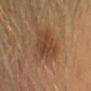Captured during whole-body skin photography for melanoma surveillance; the lesion was not biopsied. This is a cross-polarized tile. A region of skin cropped from a whole-body photographic capture, roughly 15 mm wide. A female subject, aged around 50. The recorded lesion diameter is about 6 mm. Automated tile analysis of the lesion measured a normalized lesion–skin contrast near 7. The analysis additionally found a within-lesion color-variation index near 3.5/10. And it measured a classifier nevus-likeness of about 65/100 and a detector confidence of about 100 out of 100 that the crop contains a lesion.